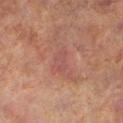{
  "biopsy_status": "not biopsied; imaged during a skin examination",
  "image": {
    "source": "total-body photography crop",
    "field_of_view_mm": 15
  },
  "lesion_size": {
    "long_diameter_mm_approx": 2.5
  },
  "lighting": "cross-polarized",
  "patient": {
    "sex": "male",
    "age_approx": 70
  },
  "site": "left lower leg"
}